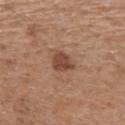{
  "biopsy_status": "not biopsied; imaged during a skin examination",
  "site": "upper back",
  "patient": {
    "sex": "male",
    "age_approx": 70
  },
  "lighting": "white-light",
  "automated_metrics": {
    "area_mm2_approx": 5.5,
    "shape_asymmetry": 0.3,
    "vs_skin_darker_L": 11.0,
    "vs_skin_contrast_norm": 8.5,
    "border_irregularity_0_10": 2.5,
    "peripheral_color_asymmetry": 0.5,
    "nevus_likeness_0_100": 85,
    "lesion_detection_confidence_0_100": 100
  },
  "image": {
    "source": "total-body photography crop",
    "field_of_view_mm": 15
  },
  "lesion_size": {
    "long_diameter_mm_approx": 3.0
  }
}